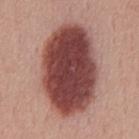{
  "biopsy_status": "not biopsied; imaged during a skin examination",
  "patient": {
    "sex": "male",
    "age_approx": 55
  },
  "image": {
    "source": "total-body photography crop",
    "field_of_view_mm": 15
  },
  "site": "mid back",
  "lesion_size": {
    "long_diameter_mm_approx": 10.5
  },
  "lighting": "white-light"
}